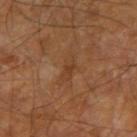No biopsy was performed on this lesion — it was imaged during a full skin examination and was not determined to be concerning.
On the right upper arm.
A roughly 15 mm field-of-view crop from a total-body skin photograph.
Approximately 3.5 mm at its widest.
An algorithmic analysis of the crop reported a shape-asymmetry score of about 0.3 (0 = symmetric). The software also gave an automated nevus-likeness rating near 0 out of 100.
A male patient, aged around 70.